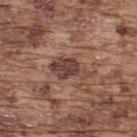biopsy status: no biopsy performed (imaged during a skin exam) | imaging modality: ~15 mm crop, total-body skin-cancer survey | patient: male, aged 73–77 | anatomic site: the back.